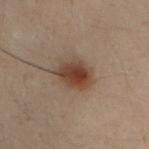Captured under cross-polarized illumination. A 15 mm close-up extracted from a 3D total-body photography capture. The patient is a male aged around 30. On the arm. Longest diameter approximately 4.5 mm.